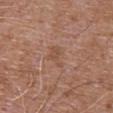Recorded during total-body skin imaging; not selected for excision or biopsy.
On the front of the torso.
The patient is a male aged approximately 75.
Automated image analysis of the tile measured a lesion color around L≈50 a*≈20 b*≈30 in CIELAB, about 6 CIELAB-L* units darker than the surrounding skin, and a lesion-to-skin contrast of about 5 (normalized; higher = more distinct). The software also gave a border-irregularity rating of about 5/10 and a peripheral color-asymmetry measure near 0.5. And it measured an automated nevus-likeness rating near 0 out of 100 and a detector confidence of about 100 out of 100 that the crop contains a lesion.
This is a white-light tile.
A close-up tile cropped from a whole-body skin photograph, about 15 mm across.
The lesion's longest dimension is about 3 mm.